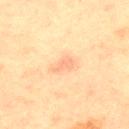{"biopsy_status": "not biopsied; imaged during a skin examination", "lesion_size": {"long_diameter_mm_approx": 3.0}, "automated_metrics": {"area_mm2_approx": 4.0, "eccentricity": 0.85, "shape_asymmetry": 0.4, "color_variation_0_10": 1.5, "peripheral_color_asymmetry": 0.5}, "site": "chest", "image": {"source": "total-body photography crop", "field_of_view_mm": 15}, "patient": {"sex": "male", "age_approx": 65}}